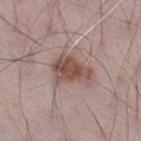follow-up: imaged on a skin check; not biopsied | imaging modality: ~15 mm crop, total-body skin-cancer survey | subject: male, roughly 70 years of age | location: the right thigh.